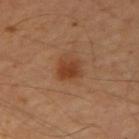workup = no biopsy performed (imaged during a skin exam)
size = about 2.5 mm
subject = male, aged 63–67
automated lesion analysis = an area of roughly 5 mm², an eccentricity of roughly 0.55, and two-axis asymmetry of about 0.2; border irregularity of about 1.5 on a 0–10 scale and a peripheral color-asymmetry measure near 1.5
image = 15 mm crop, total-body photography
body site = the right upper arm
tile lighting = cross-polarized